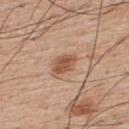Assessment:
Captured during whole-body skin photography for melanoma surveillance; the lesion was not biopsied.
Context:
The lesion is on the upper back. Longest diameter approximately 3 mm. A male subject about 55 years old. Cropped from a total-body skin-imaging series; the visible field is about 15 mm. The tile uses white-light illumination. An algorithmic analysis of the crop reported border irregularity of about 2.5 on a 0–10 scale, internal color variation of about 3 on a 0–10 scale, and radial color variation of about 1. And it measured a nevus-likeness score of about 90/100 and a detector confidence of about 100 out of 100 that the crop contains a lesion.